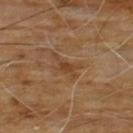- biopsy status · imaged on a skin check; not biopsied
- image · ~15 mm crop, total-body skin-cancer survey
- patient · male, aged approximately 60
- site · the chest